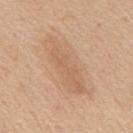* follow-up: total-body-photography surveillance lesion; no biopsy
* anatomic site: the mid back
* acquisition: ~15 mm crop, total-body skin-cancer survey
* image-analysis metrics: a lesion area of about 18 mm² and a symmetry-axis asymmetry near 0.2; an average lesion color of about L≈62 a*≈18 b*≈34 (CIELAB), a lesion–skin lightness drop of about 7, and a normalized lesion–skin contrast near 5; an automated nevus-likeness rating near 0 out of 100 and a lesion-detection confidence of about 100/100
* lesion size: ~8 mm (longest diameter)
* illumination: white-light illumination
* patient: male, aged 58 to 62